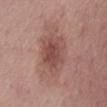{"biopsy_status": "not biopsied; imaged during a skin examination", "lesion_size": {"long_diameter_mm_approx": 5.5}, "patient": {"sex": "female", "age_approx": 50}, "site": "chest", "lighting": "white-light", "automated_metrics": {"area_mm2_approx": 16.0, "eccentricity": 0.75, "shape_asymmetry": 0.15, "border_irregularity_0_10": 2.0, "color_variation_0_10": 4.0, "peripheral_color_asymmetry": 1.5}, "image": {"source": "total-body photography crop", "field_of_view_mm": 15}}